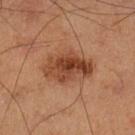This lesion was catalogued during total-body skin photography and was not selected for biopsy. About 6 mm across. On the left lower leg. A 15 mm crop from a total-body photograph taken for skin-cancer surveillance. Captured under cross-polarized illumination. A male patient aged 58 to 62. The lesion-visualizer software estimated a lesion area of about 17 mm², a shape eccentricity near 0.75, and a symmetry-axis asymmetry near 0.2. And it measured a lesion color around L≈44 a*≈24 b*≈33 in CIELAB and a lesion–skin lightness drop of about 12. It also reported border irregularity of about 2.5 on a 0–10 scale and radial color variation of about 3.